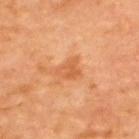site: the upper back
size: ~3 mm (longest diameter)
image source: total-body-photography crop, ~15 mm field of view
automated metrics: a mean CIELAB color near L≈59 a*≈28 b*≈43, a lesion–skin lightness drop of about 8, and a normalized border contrast of about 5.5; a classifier nevus-likeness of about 0/100
patient: aged around 65